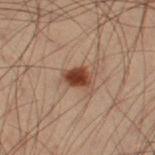workup — catalogued during a skin exam; not biopsied | image — ~15 mm tile from a whole-body skin photo | diameter — ≈3 mm | body site — the right thigh | automated lesion analysis — a lesion color around L≈32 a*≈19 b*≈25 in CIELAB, about 14 CIELAB-L* units darker than the surrounding skin, and a lesion-to-skin contrast of about 12.5 (normalized; higher = more distinct); a border-irregularity rating of about 2.5/10, a color-variation rating of about 3/10, and radial color variation of about 1 | lighting — cross-polarized illumination | patient — male, about 55 years old.